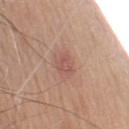Captured during whole-body skin photography for melanoma surveillance; the lesion was not biopsied. The lesion's longest dimension is about 2.5 mm. Automated tile analysis of the lesion measured an average lesion color of about L≈54 a*≈24 b*≈24 (CIELAB). The analysis additionally found a color-variation rating of about 2/10 and peripheral color asymmetry of about 1. It also reported a classifier nevus-likeness of about 10/100 and lesion-presence confidence of about 100/100. A male patient aged 58 to 62. A close-up tile cropped from a whole-body skin photograph, about 15 mm across. The lesion is located on the left upper arm.